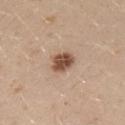Case summary:
- notes: no biopsy performed (imaged during a skin exam)
- diameter: ~2.5 mm (longest diameter)
- body site: the left upper arm
- subject: male, aged 28 to 32
- image: 15 mm crop, total-body photography
- automated metrics: an area of roughly 5.5 mm² and an outline eccentricity of about 0.4 (0 = round, 1 = elongated); a lesion color around L≈50 a*≈19 b*≈29 in CIELAB, about 16 CIELAB-L* units darker than the surrounding skin, and a normalized lesion–skin contrast near 11
- tile lighting: white-light illumination